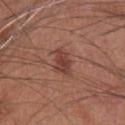This lesion was catalogued during total-body skin photography and was not selected for biopsy. An algorithmic analysis of the crop reported a border-irregularity index near 3.5/10 and internal color variation of about 2.5 on a 0–10 scale. And it measured a nevus-likeness score of about 85/100 and lesion-presence confidence of about 100/100. The subject is a male roughly 60 years of age. A lesion tile, about 15 mm wide, cut from a 3D total-body photograph. The lesion is on the chest.